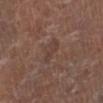This lesion was catalogued during total-body skin photography and was not selected for biopsy. Longest diameter approximately 3.5 mm. Automated tile analysis of the lesion measured an area of roughly 4.5 mm², an eccentricity of roughly 0.95, and two-axis asymmetry of about 0.25. It also reported an average lesion color of about L≈39 a*≈17 b*≈23 (CIELAB) and about 5 CIELAB-L* units darker than the surrounding skin. The software also gave a border-irregularity index near 3.5/10 and a color-variation rating of about 1/10. And it measured a lesion-detection confidence of about 100/100. This is a white-light tile. From the left lower leg. The subject is a male approximately 75 years of age. Cropped from a whole-body photographic skin survey; the tile spans about 15 mm.